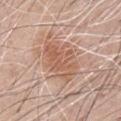No biopsy was performed on this lesion — it was imaged during a full skin examination and was not determined to be concerning. Imaged with white-light lighting. The total-body-photography lesion software estimated a shape eccentricity near 0.75 and a symmetry-axis asymmetry near 0.2. It also reported a border-irregularity index near 3/10, internal color variation of about 3.5 on a 0–10 scale, and radial color variation of about 1.5. The software also gave lesion-presence confidence of about 100/100. A male subject, aged approximately 70. The lesion's longest dimension is about 5.5 mm. Cropped from a total-body skin-imaging series; the visible field is about 15 mm. On the front of the torso.